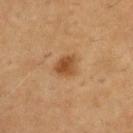Assessment:
Imaged during a routine full-body skin examination; the lesion was not biopsied and no histopathology is available.
Acquisition and patient details:
The lesion-visualizer software estimated a lesion area of about 5.5 mm², an outline eccentricity of about 0.45 (0 = round, 1 = elongated), and a symmetry-axis asymmetry near 0.3. The analysis additionally found a mean CIELAB color near L≈43 a*≈19 b*≈34, about 10 CIELAB-L* units darker than the surrounding skin, and a lesion-to-skin contrast of about 8.5 (normalized; higher = more distinct). The analysis additionally found an automated nevus-likeness rating near 100 out of 100 and a lesion-detection confidence of about 100/100. Captured under cross-polarized illumination. This image is a 15 mm lesion crop taken from a total-body photograph. The subject is a male aged 58 to 62.